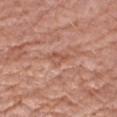Impression:
Recorded during total-body skin imaging; not selected for excision or biopsy.
Background:
A female patient, aged approximately 70. The lesion-visualizer software estimated an outline eccentricity of about 0.9 (0 = round, 1 = elongated) and a symmetry-axis asymmetry near 0.4. And it measured a lesion color around L≈54 a*≈25 b*≈30 in CIELAB, a lesion–skin lightness drop of about 7, and a lesion-to-skin contrast of about 5.5 (normalized; higher = more distinct). The software also gave a nevus-likeness score of about 0/100. Imaged with white-light lighting. The lesion is located on the right upper arm. About 2.5 mm across. Cropped from a total-body skin-imaging series; the visible field is about 15 mm.